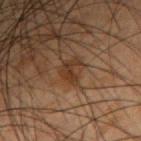Recorded during total-body skin imaging; not selected for excision or biopsy. The lesion is on the right forearm. A lesion tile, about 15 mm wide, cut from a 3D total-body photograph. Measured at roughly 3 mm in maximum diameter. A male patient, roughly 50 years of age. The lesion-visualizer software estimated an average lesion color of about L≈26 a*≈15 b*≈24 (CIELAB), about 7 CIELAB-L* units darker than the surrounding skin, and a normalized border contrast of about 7.5. The software also gave border irregularity of about 6.5 on a 0–10 scale, a within-lesion color-variation index near 2.5/10, and a peripheral color-asymmetry measure near 1. The tile uses cross-polarized illumination.